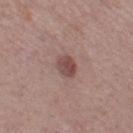biopsy status: imaged on a skin check; not biopsied.